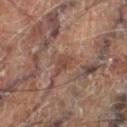The lesion was tiled from a total-body skin photograph and was not biopsied. Measured at roughly 3 mm in maximum diameter. The tile uses cross-polarized illumination. The patient is a male aged 68 to 72. This image is a 15 mm lesion crop taken from a total-body photograph. From the right thigh.